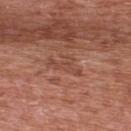lighting=white-light illumination; imaging modality=~15 mm crop, total-body skin-cancer survey; patient=male, roughly 70 years of age; body site=the upper back; lesion diameter=about 4 mm.